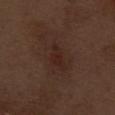Q: Was a biopsy performed?
A: catalogued during a skin exam; not biopsied
Q: How was the tile lit?
A: white-light illumination
Q: What are the patient's age and sex?
A: male, aged 68 to 72
Q: What kind of image is this?
A: ~15 mm crop, total-body skin-cancer survey
Q: Lesion location?
A: the right thigh
Q: What did automated image analysis measure?
A: a lesion color around L≈23 a*≈18 b*≈22 in CIELAB, a lesion–skin lightness drop of about 5, and a normalized border contrast of about 6.5